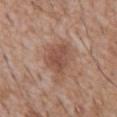Clinical impression:
Part of a total-body skin-imaging series; this lesion was reviewed on a skin check and was not flagged for biopsy.
Background:
The lesion is on the front of the torso. The subject is a male in their 60s. A roughly 15 mm field-of-view crop from a total-body skin photograph.